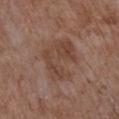follow-up: imaged on a skin check; not biopsied | image: 15 mm crop, total-body photography | tile lighting: white-light | site: the chest | diameter: about 5.5 mm | TBP lesion metrics: a mean CIELAB color near L≈43 a*≈19 b*≈26, about 7 CIELAB-L* units darker than the surrounding skin, and a lesion-to-skin contrast of about 6 (normalized; higher = more distinct); a detector confidence of about 100 out of 100 that the crop contains a lesion | patient: female, aged around 80.